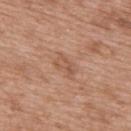biopsy_status: not biopsied; imaged during a skin examination
site: mid back
image:
  source: total-body photography crop
  field_of_view_mm: 15
lesion_size:
  long_diameter_mm_approx: 3.0
patient:
  sex: male
  age_approx: 50
lighting: white-light